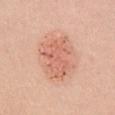biopsy status=total-body-photography surveillance lesion; no biopsy
subject=female, aged 58 to 62
tile lighting=white-light illumination
lesion diameter=about 5.5 mm
location=the chest
automated metrics=a nevus-likeness score of about 95/100 and a detector confidence of about 100 out of 100 that the crop contains a lesion
acquisition=total-body-photography crop, ~15 mm field of view